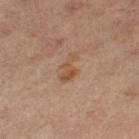No biopsy was performed on this lesion — it was imaged during a full skin examination and was not determined to be concerning.